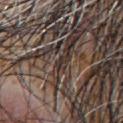The lesion was photographed on a routine skin check and not biopsied; there is no pathology result.
Imaged with white-light lighting.
The lesion-visualizer software estimated a lesion color around L≈31 a*≈11 b*≈17 in CIELAB, a lesion–skin lightness drop of about 9, and a normalized border contrast of about 9.5. The software also gave border irregularity of about 2.5 on a 0–10 scale and a peripheral color-asymmetry measure near 0. The software also gave a classifier nevus-likeness of about 0/100 and a detector confidence of about 0 out of 100 that the crop contains a lesion.
Measured at roughly 1.5 mm in maximum diameter.
From the chest.
A male patient, aged 53–57.
Cropped from a whole-body photographic skin survey; the tile spans about 15 mm.